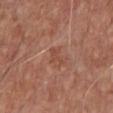Notes:
* follow-up · total-body-photography surveillance lesion; no biopsy
* TBP lesion metrics · a footprint of about 3 mm², a shape eccentricity near 0.8, and two-axis asymmetry of about 0.55; a color-variation rating of about 0/10
* body site · the chest
* lesion size · ≈2.5 mm
* tile lighting · white-light
* subject · male, aged 63 to 67
* acquisition · ~15 mm crop, total-body skin-cancer survey